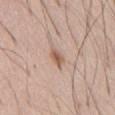The lesion was photographed on a routine skin check and not biopsied; there is no pathology result. The lesion is on the abdomen. The subject is a male aged approximately 60. The lesion's longest dimension is about 2.5 mm. Captured under white-light illumination. A 15 mm close-up extracted from a 3D total-body photography capture. The lesion-visualizer software estimated a shape eccentricity near 0.85 and two-axis asymmetry of about 0.25. The analysis additionally found a border-irregularity rating of about 2.5/10 and a peripheral color-asymmetry measure near 0.5.